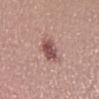Acquisition and patient details: A 15 mm crop from a total-body photograph taken for skin-cancer surveillance. About 3.5 mm across. On the left lower leg. A female subject, aged 23 to 27.